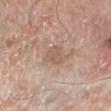<record>
  <biopsy_status>not biopsied; imaged during a skin examination</biopsy_status>
  <image>
    <source>total-body photography crop</source>
    <field_of_view_mm>15</field_of_view_mm>
  </image>
  <site>left lower leg</site>
  <patient>
    <sex>male</sex>
    <age_approx>80</age_approx>
  </patient>
</record>